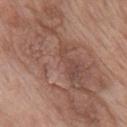Findings:
– follow-up: no biopsy performed (imaged during a skin exam)
– lesion diameter: ~17.5 mm (longest diameter)
– imaging modality: 15 mm crop, total-body photography
– site: the chest
– patient: female, aged 58–62
– automated lesion analysis: a lesion area of about 95 mm² and a shape-asymmetry score of about 0.35 (0 = symmetric); a lesion color around L≈51 a*≈19 b*≈26 in CIELAB, about 9 CIELAB-L* units darker than the surrounding skin, and a normalized border contrast of about 6; border irregularity of about 7 on a 0–10 scale, a within-lesion color-variation index near 6/10, and peripheral color asymmetry of about 2.5; a lesion-detection confidence of about 80/100
– tile lighting: white-light